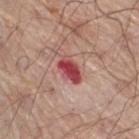Recorded during total-body skin imaging; not selected for excision or biopsy. A male subject, roughly 70 years of age. From the right thigh. A close-up tile cropped from a whole-body skin photograph, about 15 mm across.